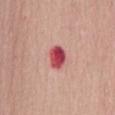lighting: white-light
site: mid back
automated_metrics:
  eccentricity: 0.75
  cielab_L: 50
  cielab_a: 39
  cielab_b: 24
  vs_skin_contrast_norm: 11.5
  border_irregularity_0_10: 1.5
  color_variation_0_10: 6.0
  peripheral_color_asymmetry: 2.0
  nevus_likeness_0_100: 0
  lesion_detection_confidence_0_100: 100
lesion_size:
  long_diameter_mm_approx: 3.0
patient:
  sex: female
  age_approx: 60
image:
  source: total-body photography crop
  field_of_view_mm: 15